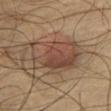Notes:
* follow-up — catalogued during a skin exam; not biopsied